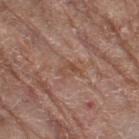workup = imaged on a skin check; not biopsied